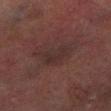  biopsy_status: not biopsied; imaged during a skin examination
  site: right lower leg
  lighting: cross-polarized
  lesion_size:
    long_diameter_mm_approx: 4.0
  patient:
    sex: male
    age_approx: 75
  image:
    source: total-body photography crop
    field_of_view_mm: 15